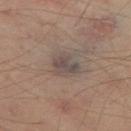Q: Was a biopsy performed?
A: no biopsy performed (imaged during a skin exam)
Q: What are the patient's age and sex?
A: aged around 55
Q: How was this image acquired?
A: 15 mm crop, total-body photography
Q: Lesion location?
A: the left thigh
Q: What lighting was used for the tile?
A: cross-polarized illumination